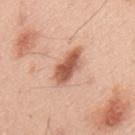Captured during whole-body skin photography for melanoma surveillance; the lesion was not biopsied.
The lesion-visualizer software estimated about 15 CIELAB-L* units darker than the surrounding skin and a normalized border contrast of about 9.5.
Measured at roughly 5 mm in maximum diameter.
The lesion is on the mid back.
Imaged with white-light lighting.
Cropped from a total-body skin-imaging series; the visible field is about 15 mm.
A male patient, in their mid- to late 40s.